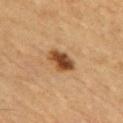biopsy status — imaged on a skin check; not biopsied | subject — male, in their mid- to late 50s | anatomic site — the chest | image source — total-body-photography crop, ~15 mm field of view | lighting — cross-polarized.